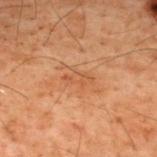biopsy status: no biopsy performed (imaged during a skin exam); patient: male, aged 58 to 62; location: the upper back; illumination: cross-polarized; image: 15 mm crop, total-body photography; TBP lesion metrics: a lesion area of about 3 mm², an outline eccentricity of about 0.9 (0 = round, 1 = elongated), and a shape-asymmetry score of about 0.45 (0 = symmetric).